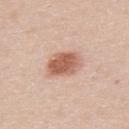biopsy status: imaged on a skin check; not biopsied
illumination: white-light illumination
acquisition: total-body-photography crop, ~15 mm field of view
automated lesion analysis: a lesion area of about 10 mm², a shape eccentricity near 0.75, and a shape-asymmetry score of about 0.2 (0 = symmetric)
body site: the back
diameter: ~4 mm (longest diameter)
patient: male, aged 38–42